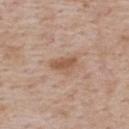Findings:
• follow-up: catalogued during a skin exam; not biopsied
• anatomic site: the upper back
• automated metrics: a lesion-detection confidence of about 100/100
• image: ~15 mm crop, total-body skin-cancer survey
• tile lighting: white-light illumination
• subject: male, roughly 75 years of age